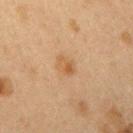Imaged during a routine full-body skin examination; the lesion was not biopsied and no histopathology is available. Imaged with cross-polarized lighting. The patient is a female aged 38 to 42. A lesion tile, about 15 mm wide, cut from a 3D total-body photograph. Approximately 2.5 mm at its widest. The lesion-visualizer software estimated a lesion-to-skin contrast of about 6 (normalized; higher = more distinct). The lesion is on the right upper arm.